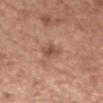biopsy status: catalogued during a skin exam; not biopsied
lesion diameter: about 2.5 mm
image source: 15 mm crop, total-body photography
automated lesion analysis: a lesion area of about 4.5 mm², a shape eccentricity near 0.7, and a symmetry-axis asymmetry near 0.3; a color-variation rating of about 2.5/10 and peripheral color asymmetry of about 1; a lesion-detection confidence of about 100/100
patient: female, aged 68–72
anatomic site: the head or neck